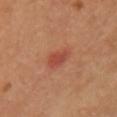biopsy status = imaged on a skin check; not biopsied
patient = female, in their 50s
lesion diameter = ~3 mm (longest diameter)
site = the front of the torso
imaging modality = 15 mm crop, total-body photography
automated lesion analysis = an area of roughly 5 mm² and a shape eccentricity near 0.85; a border-irregularity index near 2.5/10 and radial color variation of about 0.5; a detector confidence of about 100 out of 100 that the crop contains a lesion
tile lighting = cross-polarized